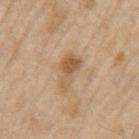Impression: Recorded during total-body skin imaging; not selected for excision or biopsy. Background: About 4 mm across. A roughly 15 mm field-of-view crop from a total-body skin photograph. The patient is a male aged 68 to 72. The lesion is located on the arm.